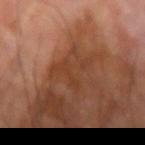workup: imaged on a skin check; not biopsied
automated lesion analysis: a border-irregularity index near 9.5/10, internal color variation of about 3 on a 0–10 scale, and radial color variation of about 1
anatomic site: the arm
patient: male, aged approximately 70
tile lighting: cross-polarized illumination
image: total-body-photography crop, ~15 mm field of view